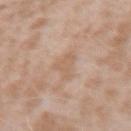Q: Was this lesion biopsied?
A: imaged on a skin check; not biopsied
Q: What lighting was used for the tile?
A: white-light illumination
Q: How was this image acquired?
A: ~15 mm tile from a whole-body skin photo
Q: What did automated image analysis measure?
A: a lesion area of about 4.5 mm², an eccentricity of roughly 0.7, and a shape-asymmetry score of about 0.6 (0 = symmetric); an average lesion color of about L≈61 a*≈17 b*≈30 (CIELAB), about 6 CIELAB-L* units darker than the surrounding skin, and a normalized border contrast of about 4.5; a nevus-likeness score of about 0/100
Q: What is the lesion's diameter?
A: ≈3 mm
Q: What is the anatomic site?
A: the right forearm
Q: Patient demographics?
A: female, in their mid-20s